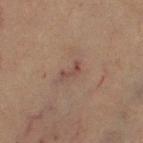{
  "biopsy_status": "not biopsied; imaged during a skin examination"
}